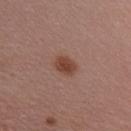Recorded during total-body skin imaging; not selected for excision or biopsy. A region of skin cropped from a whole-body photographic capture, roughly 15 mm wide. Measured at roughly 2.5 mm in maximum diameter. On the right upper arm. The patient is a female in their mid- to late 50s.